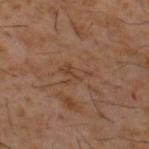follow-up=total-body-photography surveillance lesion; no biopsy
patient=male, aged 58 to 62
image source=~15 mm crop, total-body skin-cancer survey
anatomic site=the upper back
tile lighting=cross-polarized illumination
size=about 3.5 mm